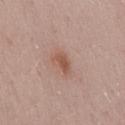biopsy_status: not biopsied; imaged during a skin examination
patient:
  sex: male
  age_approx: 55
lesion_size:
  long_diameter_mm_approx: 3.0
site: mid back
image:
  source: total-body photography crop
  field_of_view_mm: 15